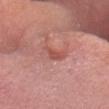Notes:
- notes: total-body-photography surveillance lesion; no biopsy
- imaging modality: 15 mm crop, total-body photography
- patient: male, aged 53–57
- body site: the head or neck
- size: about 2.5 mm
- image-analysis metrics: a border-irregularity index near 4/10, a color-variation rating of about 0.5/10, and radial color variation of about 0; a nevus-likeness score of about 5/100 and a detector confidence of about 85 out of 100 that the crop contains a lesion
- illumination: white-light illumination